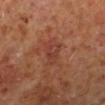No biopsy was performed on this lesion — it was imaged during a full skin examination and was not determined to be concerning.
A male subject roughly 60 years of age.
A 15 mm crop from a total-body photograph taken for skin-cancer surveillance.
The lesion is on the left lower leg.